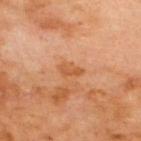From the upper back. The patient is a male aged 68 to 72. A close-up tile cropped from a whole-body skin photograph, about 15 mm across.